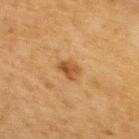Part of a total-body skin-imaging series; this lesion was reviewed on a skin check and was not flagged for biopsy.
Cropped from a total-body skin-imaging series; the visible field is about 15 mm.
Approximately 2.5 mm at its widest.
Located on the back.
A female subject, aged approximately 60.
An algorithmic analysis of the crop reported roughly 9 lightness units darker than nearby skin and a normalized border contrast of about 7.5. It also reported a border-irregularity index near 2/10, a within-lesion color-variation index near 3.5/10, and peripheral color asymmetry of about 1.5.